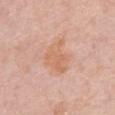| feature | finding |
|---|---|
| notes | catalogued during a skin exam; not biopsied |
| anatomic site | the chest |
| TBP lesion metrics | an area of roughly 11 mm², an eccentricity of roughly 0.65, and a shape-asymmetry score of about 0.4 (0 = symmetric); a lesion color around L≈65 a*≈23 b*≈33 in CIELAB and a lesion–skin lightness drop of about 7; border irregularity of about 4.5 on a 0–10 scale, internal color variation of about 2.5 on a 0–10 scale, and peripheral color asymmetry of about 1; an automated nevus-likeness rating near 0 out of 100 and a lesion-detection confidence of about 100/100 |
| patient | female, about 75 years old |
| image source | 15 mm crop, total-body photography |
| lesion diameter | about 4.5 mm |
| tile lighting | white-light illumination |